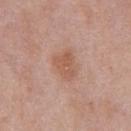Case summary:
- workup · total-body-photography surveillance lesion; no biopsy
- lighting · white-light illumination
- subject · male, in their mid- to late 50s
- acquisition · ~15 mm tile from a whole-body skin photo
- body site · the chest
- TBP lesion metrics · an area of roughly 6 mm², an eccentricity of roughly 0.65, and two-axis asymmetry of about 0.35; a mean CIELAB color near L≈56 a*≈22 b*≈30, a lesion–skin lightness drop of about 8, and a lesion-to-skin contrast of about 6.5 (normalized; higher = more distinct)
- size · ~3 mm (longest diameter)